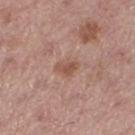The lesion was photographed on a routine skin check and not biopsied; there is no pathology result. A lesion tile, about 15 mm wide, cut from a 3D total-body photograph. The patient is a male aged approximately 55. On the right thigh. This is a white-light tile.